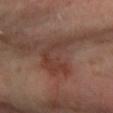follow-up: no biopsy performed (imaged during a skin exam)
body site: the arm
subject: female, in their mid-50s
acquisition: total-body-photography crop, ~15 mm field of view